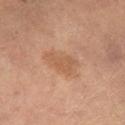follow-up = imaged on a skin check; not biopsied | acquisition = 15 mm crop, total-body photography | lighting = cross-polarized illumination | subject = male, in their mid-60s.